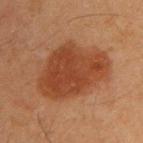Clinical impression:
Captured during whole-body skin photography for melanoma surveillance; the lesion was not biopsied.
Background:
Located on the upper back. Captured under cross-polarized illumination. The recorded lesion diameter is about 8 mm. A male subject, approximately 45 years of age. Cropped from a total-body skin-imaging series; the visible field is about 15 mm.